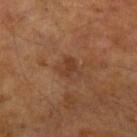Clinical impression:
No biopsy was performed on this lesion — it was imaged during a full skin examination and was not determined to be concerning.
Context:
The patient is a male aged approximately 65. From the arm. The tile uses cross-polarized illumination. A close-up tile cropped from a whole-body skin photograph, about 15 mm across.